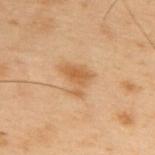The lesion was photographed on a routine skin check and not biopsied; there is no pathology result. A male subject, aged approximately 55. The lesion is on the upper back. This image is a 15 mm lesion crop taken from a total-body photograph.